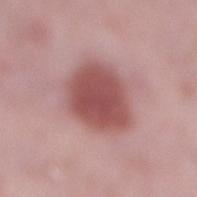This image is a 15 mm lesion crop taken from a total-body photograph.
A female subject aged around 40.
Automated image analysis of the tile measured a lesion area of about 22 mm² and an outline eccentricity of about 0.4 (0 = round, 1 = elongated). It also reported a mean CIELAB color near L≈51 a*≈26 b*≈22, roughly 14 lightness units darker than nearby skin, and a normalized border contrast of about 9.5. The software also gave a border-irregularity index near 1.5/10, a color-variation rating of about 3/10, and radial color variation of about 1. It also reported a lesion-detection confidence of about 100/100.
Located on the right lower leg.
The lesion's longest dimension is about 6 mm.